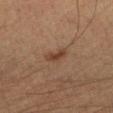No biopsy was performed on this lesion — it was imaged during a full skin examination and was not determined to be concerning. The lesion is on the left upper arm. Approximately 3 mm at its widest. A male subject, approximately 55 years of age. Captured under cross-polarized illumination. A close-up tile cropped from a whole-body skin photograph, about 15 mm across. Automated image analysis of the tile measured two-axis asymmetry of about 0.35. The analysis additionally found an average lesion color of about L≈35 a*≈17 b*≈26 (CIELAB), a lesion–skin lightness drop of about 8, and a normalized lesion–skin contrast near 7.5. The software also gave border irregularity of about 3.5 on a 0–10 scale, internal color variation of about 1.5 on a 0–10 scale, and a peripheral color-asymmetry measure near 0.5.